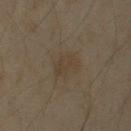Cropped from a whole-body photographic skin survey; the tile spans about 15 mm.
About 3 mm across.
On the right upper arm.
A male patient, aged 58 to 62.
This is a cross-polarized tile.